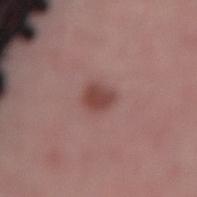Recorded during total-body skin imaging; not selected for excision or biopsy. A female patient, about 45 years old. The lesion is located on the back. Cropped from a whole-body photographic skin survey; the tile spans about 15 mm.